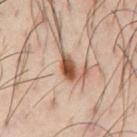workup: imaged on a skin check; not biopsied
patient: male, aged approximately 40
size: ≈3 mm
imaging modality: 15 mm crop, total-body photography
body site: the chest
lighting: cross-polarized